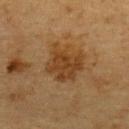A male subject, about 85 years old or older. A lesion tile, about 15 mm wide, cut from a 3D total-body photograph. This is a cross-polarized tile. Automated image analysis of the tile measured a footprint of about 14 mm², an eccentricity of roughly 0.3, and two-axis asymmetry of about 0.25. The software also gave an average lesion color of about L≈35 a*≈17 b*≈33 (CIELAB) and a lesion-to-skin contrast of about 7.5 (normalized; higher = more distinct). The analysis additionally found a classifier nevus-likeness of about 45/100. The lesion is located on the upper back. Approximately 4.5 mm at its widest.